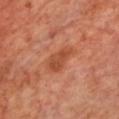<lesion>
  <image>
    <source>total-body photography crop</source>
    <field_of_view_mm>15</field_of_view_mm>
  </image>
  <site>chest</site>
  <patient>
    <sex>male</sex>
    <age_approx>70</age_approx>
  </patient>
  <lighting>cross-polarized</lighting>
  <lesion_size>
    <long_diameter_mm_approx>3.5</long_diameter_mm_approx>
  </lesion_size>
</lesion>